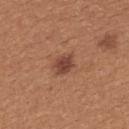No biopsy was performed on this lesion — it was imaged during a full skin examination and was not determined to be concerning. A region of skin cropped from a whole-body photographic capture, roughly 15 mm wide. Captured under white-light illumination. A female patient in their 40s. Automated tile analysis of the lesion measured a lesion color around L≈43 a*≈23 b*≈28 in CIELAB, roughly 11 lightness units darker than nearby skin, and a normalized lesion–skin contrast near 9. It also reported a border-irregularity rating of about 2/10, a within-lesion color-variation index near 2.5/10, and peripheral color asymmetry of about 1. The software also gave a classifier nevus-likeness of about 75/100 and a detector confidence of about 100 out of 100 that the crop contains a lesion. From the back.